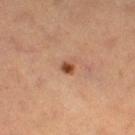No biopsy was performed on this lesion — it was imaged during a full skin examination and was not determined to be concerning. From the right thigh. A female subject, approximately 65 years of age. This image is a 15 mm lesion crop taken from a total-body photograph. Measured at roughly 1.5 mm in maximum diameter. This is a cross-polarized tile.